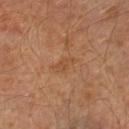- location — the left lower leg
- imaging modality — ~15 mm tile from a whole-body skin photo
- subject — male, roughly 60 years of age